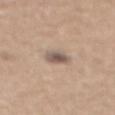• biopsy status — no biopsy performed (imaged during a skin exam)
• patient — male, aged 63 to 67
• illumination — white-light illumination
• anatomic site — the back
• acquisition — ~15 mm tile from a whole-body skin photo